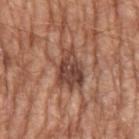Impression: No biopsy was performed on this lesion — it was imaged during a full skin examination and was not determined to be concerning. Context: This is a white-light tile. A male subject, roughly 65 years of age. The total-body-photography lesion software estimated a lesion color around L≈45 a*≈22 b*≈27 in CIELAB, about 12 CIELAB-L* units darker than the surrounding skin, and a lesion-to-skin contrast of about 9 (normalized; higher = more distinct). The software also gave a border-irregularity rating of about 4.5/10 and a peripheral color-asymmetry measure near 2. The lesion is located on the left upper arm. A close-up tile cropped from a whole-body skin photograph, about 15 mm across.